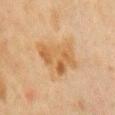Impression:
The lesion was tiled from a total-body skin photograph and was not biopsied.
Background:
The lesion-visualizer software estimated an area of roughly 15 mm², an eccentricity of roughly 0.8, and a shape-asymmetry score of about 0.4 (0 = symmetric). It also reported border irregularity of about 4.5 on a 0–10 scale, internal color variation of about 5 on a 0–10 scale, and peripheral color asymmetry of about 1.5. Imaged with cross-polarized lighting. Located on the chest. A female subject, roughly 50 years of age. A 15 mm crop from a total-body photograph taken for skin-cancer surveillance.